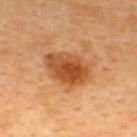{"biopsy_status": "not biopsied; imaged during a skin examination", "image": {"source": "total-body photography crop", "field_of_view_mm": 15}, "site": "upper back", "patient": {"sex": "male", "age_approx": 45}}